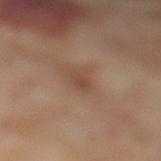{"biopsy_status": "not biopsied; imaged during a skin examination", "image": {"source": "total-body photography crop", "field_of_view_mm": 15}, "automated_metrics": {"eccentricity": 0.8, "cielab_L": 47, "cielab_a": 18, "cielab_b": 29, "vs_skin_darker_L": 7.0, "vs_skin_contrast_norm": 6.0, "nevus_likeness_0_100": 5, "lesion_detection_confidence_0_100": 100}, "site": "left lower leg", "patient": {"sex": "female", "age_approx": 55}, "lesion_size": {"long_diameter_mm_approx": 2.5}, "lighting": "cross-polarized"}